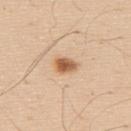Recorded during total-body skin imaging; not selected for excision or biopsy. A male subject aged 58–62. Approximately 2.5 mm at its widest. On the upper back. Imaged with white-light lighting. This image is a 15 mm lesion crop taken from a total-body photograph.